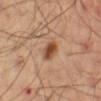  lighting: cross-polarized
  automated_metrics:
    cielab_L: 48
    cielab_a: 23
    cielab_b: 34
    vs_skin_darker_L: 14.0
    vs_skin_contrast_norm: 10.0
    nevus_likeness_0_100: 95
    lesion_detection_confidence_0_100: 100
  lesion_size:
    long_diameter_mm_approx: 2.5
  image:
    source: total-body photography crop
    field_of_view_mm: 15
  patient:
    sex: male
    age_approx: 65
  site: right thigh